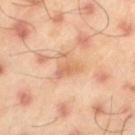Clinical impression:
No biopsy was performed on this lesion — it was imaged during a full skin examination and was not determined to be concerning.
Clinical summary:
The lesion is on the left thigh. The subject is a male in their mid-40s. This is a cross-polarized tile. Approximately 3.5 mm at its widest. A close-up tile cropped from a whole-body skin photograph, about 15 mm across.